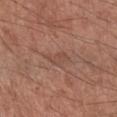This lesion was catalogued during total-body skin photography and was not selected for biopsy.
A male patient, in their mid-50s.
From the right forearm.
A 15 mm close-up tile from a total-body photography series done for melanoma screening.
About 3 mm across.